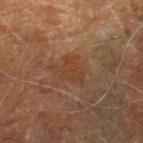Image and clinical context:
The lesion is located on the leg. A male subject, approximately 70 years of age. Cropped from a total-body skin-imaging series; the visible field is about 15 mm. Captured under cross-polarized illumination. Automated tile analysis of the lesion measured a mean CIELAB color near L≈40 a*≈20 b*≈31, a lesion–skin lightness drop of about 5, and a lesion-to-skin contrast of about 5 (normalized; higher = more distinct).